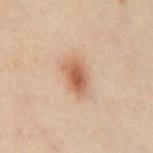Assessment: The lesion was tiled from a total-body skin photograph and was not biopsied. Context: A female patient, about 30 years old. The tile uses cross-polarized illumination. An algorithmic analysis of the crop reported a lesion area of about 7.5 mm², a shape eccentricity near 0.75, and two-axis asymmetry of about 0.15. The analysis additionally found border irregularity of about 2 on a 0–10 scale and internal color variation of about 4 on a 0–10 scale. About 4 mm across. The lesion is on the chest. A lesion tile, about 15 mm wide, cut from a 3D total-body photograph.